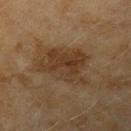Clinical impression:
No biopsy was performed on this lesion — it was imaged during a full skin examination and was not determined to be concerning.
Image and clinical context:
A 15 mm crop from a total-body photograph taken for skin-cancer surveillance. The lesion is on the left upper arm. A female subject in their 60s.